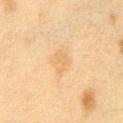The lesion was tiled from a total-body skin photograph and was not biopsied.